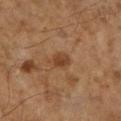Impression:
Recorded during total-body skin imaging; not selected for excision or biopsy.
Clinical summary:
Captured under cross-polarized illumination. A male subject, about 60 years old. Located on the left lower leg. Cropped from a total-body skin-imaging series; the visible field is about 15 mm. The recorded lesion diameter is about 3 mm.